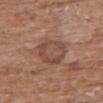follow-up = total-body-photography surveillance lesion; no biopsy
image = total-body-photography crop, ~15 mm field of view
patient = female, about 75 years old
anatomic site = the upper back
lesion diameter = ~4.5 mm (longest diameter)
illumination = white-light illumination